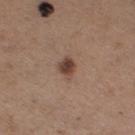Impression:
The lesion was tiled from a total-body skin photograph and was not biopsied.
Image and clinical context:
Approximately 3 mm at its widest. The lesion-visualizer software estimated a footprint of about 4.5 mm², an eccentricity of roughly 0.7, and a shape-asymmetry score of about 0.3 (0 = symmetric). The software also gave an average lesion color of about L≈43 a*≈18 b*≈25 (CIELAB) and a normalized lesion–skin contrast near 9.5. The lesion is on the right thigh. A 15 mm crop from a total-body photograph taken for skin-cancer surveillance. A female patient, aged approximately 30.